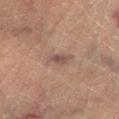Clinical impression: Captured during whole-body skin photography for melanoma surveillance; the lesion was not biopsied. Acquisition and patient details: The lesion-visualizer software estimated an average lesion color of about L≈42 a*≈16 b*≈20 (CIELAB), roughly 8 lightness units darker than nearby skin, and a normalized border contrast of about 7. And it measured a nevus-likeness score of about 0/100 and a detector confidence of about 100 out of 100 that the crop contains a lesion. The tile uses cross-polarized illumination. The subject is a male aged approximately 85 or older. Located on the left lower leg. A close-up tile cropped from a whole-body skin photograph, about 15 mm across.